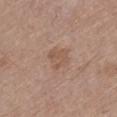notes: catalogued during a skin exam; not biopsied | illumination: white-light illumination | image source: ~15 mm crop, total-body skin-cancer survey | subject: female, approximately 55 years of age | lesion diameter: about 3 mm | location: the left lower leg | automated lesion analysis: roughly 6 lightness units darker than nearby skin.